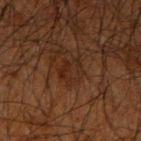No biopsy was performed on this lesion — it was imaged during a full skin examination and was not determined to be concerning. Cropped from a whole-body photographic skin survey; the tile spans about 15 mm. A male subject aged 63 to 67. On the right upper arm.